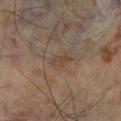Findings:
– notes — total-body-photography surveillance lesion; no biopsy
– location — the leg
– subject — male, aged 63–67
– image — ~15 mm tile from a whole-body skin photo